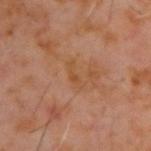<tbp_lesion>
<biopsy_status>not biopsied; imaged during a skin examination</biopsy_status>
<lesion_size>
  <long_diameter_mm_approx>3.0</long_diameter_mm_approx>
</lesion_size>
<patient>
  <sex>male</sex>
  <age_approx>60</age_approx>
</patient>
<image>
  <source>total-body photography crop</source>
  <field_of_view_mm>15</field_of_view_mm>
</image>
<site>back</site>
</tbp_lesion>